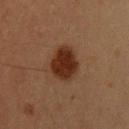Recorded during total-body skin imaging; not selected for excision or biopsy. The subject is a male aged approximately 35. Cropped from a whole-body photographic skin survey; the tile spans about 15 mm. The recorded lesion diameter is about 4 mm. Located on the head or neck. Captured under cross-polarized illumination.